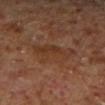notes: total-body-photography surveillance lesion; no biopsy
diameter: ~5 mm (longest diameter)
image: ~15 mm crop, total-body skin-cancer survey
anatomic site: the right lower leg
automated lesion analysis: a shape-asymmetry score of about 0.35 (0 = symmetric); a lesion–skin lightness drop of about 5 and a normalized border contrast of about 5.5; a border-irregularity rating of about 4/10, internal color variation of about 3.5 on a 0–10 scale, and radial color variation of about 1
patient: male, about 60 years old
tile lighting: cross-polarized illumination